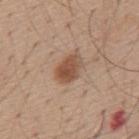workup: catalogued during a skin exam; not biopsied
site: the mid back
size: about 3.5 mm
acquisition: 15 mm crop, total-body photography
patient: male, about 55 years old